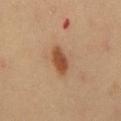follow-up: total-body-photography surveillance lesion; no biopsy | site: the front of the torso | automated metrics: a border-irregularity index near 2.5/10 and a color-variation rating of about 2.5/10 | diameter: about 4 mm | patient: male, aged 38 to 42 | imaging modality: ~15 mm crop, total-body skin-cancer survey.